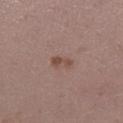Q: Was a biopsy performed?
A: imaged on a skin check; not biopsied
Q: How was this image acquired?
A: total-body-photography crop, ~15 mm field of view
Q: Lesion size?
A: about 3 mm
Q: What did automated image analysis measure?
A: an area of roughly 2.5 mm², a shape eccentricity near 0.9, and two-axis asymmetry of about 0.35; border irregularity of about 4.5 on a 0–10 scale, internal color variation of about 0 on a 0–10 scale, and peripheral color asymmetry of about 0
Q: Where on the body is the lesion?
A: the left lower leg
Q: How was the tile lit?
A: white-light illumination
Q: Patient demographics?
A: female, aged around 30